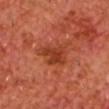follow-up: total-body-photography surveillance lesion; no biopsy | subject: male, approximately 65 years of age | image source: 15 mm crop, total-body photography.